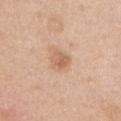follow-up = imaged on a skin check; not biopsied | image = total-body-photography crop, ~15 mm field of view | lighting = white-light | lesion diameter = ≈2.5 mm | subject = female, about 65 years old | automated lesion analysis = an eccentricity of roughly 0.55; border irregularity of about 2.5 on a 0–10 scale and a color-variation rating of about 3.5/10; an automated nevus-likeness rating near 40 out of 100 and lesion-presence confidence of about 100/100 | body site = the abdomen.